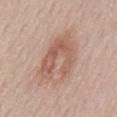Case summary:
– biopsy status — total-body-photography surveillance lesion; no biopsy
– patient — female, in their mid- to late 50s
– lighting — white-light
– lesion diameter — ~6 mm (longest diameter)
– imaging modality — ~15 mm crop, total-body skin-cancer survey
– location — the mid back